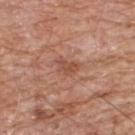<case>
  <biopsy_status>not biopsied; imaged during a skin examination</biopsy_status>
  <lighting>white-light</lighting>
  <site>upper back</site>
  <lesion_size>
    <long_diameter_mm_approx>2.5</long_diameter_mm_approx>
  </lesion_size>
  <automated_metrics>
    <cielab_L>50</cielab_L>
    <cielab_a>24</cielab_a>
    <cielab_b>31</cielab_b>
    <vs_skin_darker_L>8.0</vs_skin_darker_L>
    <vs_skin_contrast_norm>6.5</vs_skin_contrast_norm>
    <nevus_likeness_0_100>0</nevus_likeness_0_100>
    <lesion_detection_confidence_0_100>100</lesion_detection_confidence_0_100>
  </automated_metrics>
  <patient>
    <sex>male</sex>
    <age_approx>80</age_approx>
  </patient>
  <image>
    <source>total-body photography crop</source>
    <field_of_view_mm>15</field_of_view_mm>
  </image>
</case>